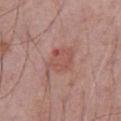The lesion was photographed on a routine skin check and not biopsied; there is no pathology result. A 15 mm crop from a total-body photograph taken for skin-cancer surveillance. A male subject about 70 years old. The lesion is on the abdomen. Measured at roughly 4 mm in maximum diameter. The tile uses white-light illumination.